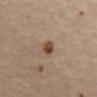The lesion is located on the chest. A female patient aged approximately 60. Captured under cross-polarized illumination. Longest diameter approximately 2.5 mm. A 15 mm close-up tile from a total-body photography series done for melanoma screening.